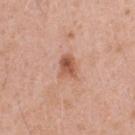The tile uses white-light illumination. A 15 mm close-up extracted from a 3D total-body photography capture. The subject is a male aged around 55. The lesion is on the chest. The lesion's longest dimension is about 3 mm.